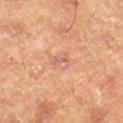Notes:
* follow-up: no biopsy performed (imaged during a skin exam)
* lighting: cross-polarized illumination
* image source: ~15 mm tile from a whole-body skin photo
* lesion size: ≈3 mm
* patient: male, in their mid- to late 40s
* image-analysis metrics: a classifier nevus-likeness of about 0/100 and lesion-presence confidence of about 100/100
* body site: the right lower leg